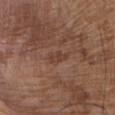Assessment:
Imaged during a routine full-body skin examination; the lesion was not biopsied and no histopathology is available.
Clinical summary:
A male patient aged around 75. The total-body-photography lesion software estimated a nevus-likeness score of about 0/100 and a detector confidence of about 100 out of 100 that the crop contains a lesion. On the front of the torso. Cropped from a total-body skin-imaging series; the visible field is about 15 mm.